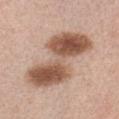Captured during whole-body skin photography for melanoma surveillance; the lesion was not biopsied.
Captured under white-light illumination.
Located on the chest.
A close-up tile cropped from a whole-body skin photograph, about 15 mm across.
The patient is a female aged approximately 40.
Approximately 9 mm at its widest.